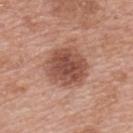Notes:
* biopsy status: total-body-photography surveillance lesion; no biopsy
* TBP lesion metrics: a mean CIELAB color near L≈50 a*≈23 b*≈28, a lesion–skin lightness drop of about 13, and a normalized border contrast of about 9
* lesion diameter: about 5 mm
* illumination: white-light
* patient: female, about 60 years old
* body site: the upper back
* imaging modality: ~15 mm crop, total-body skin-cancer survey